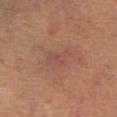Assessment: Part of a total-body skin-imaging series; this lesion was reviewed on a skin check and was not flagged for biopsy. Clinical summary: Located on the chest. Automated image analysis of the tile measured a lesion color around L≈50 a*≈22 b*≈26 in CIELAB, about 5 CIELAB-L* units darker than the surrounding skin, and a lesion-to-skin contrast of about 5 (normalized; higher = more distinct). The software also gave a color-variation rating of about 2.5/10 and radial color variation of about 1. The software also gave a nevus-likeness score of about 0/100 and a detector confidence of about 100 out of 100 that the crop contains a lesion. A lesion tile, about 15 mm wide, cut from a 3D total-body photograph. A female subject aged 48 to 52.